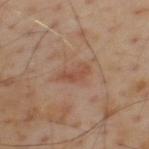No biopsy was performed on this lesion — it was imaged during a full skin examination and was not determined to be concerning.
A male patient in their mid- to late 50s.
Automated tile analysis of the lesion measured a border-irregularity rating of about 4/10, internal color variation of about 2 on a 0–10 scale, and a peripheral color-asymmetry measure near 0.5.
Captured under cross-polarized illumination.
A roughly 15 mm field-of-view crop from a total-body skin photograph.
The lesion is on the upper back.